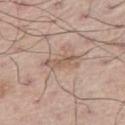Image and clinical context:
This is a white-light tile. About 4 mm across. A 15 mm close-up extracted from a 3D total-body photography capture. The patient is a male roughly 70 years of age. On the left leg. The lesion-visualizer software estimated a lesion color around L≈57 a*≈16 b*≈27 in CIELAB and about 9 CIELAB-L* units darker than the surrounding skin. It also reported border irregularity of about 5 on a 0–10 scale, internal color variation of about 0 on a 0–10 scale, and peripheral color asymmetry of about 0. And it measured a classifier nevus-likeness of about 0/100 and a lesion-detection confidence of about 85/100.